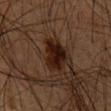Q: How was this image acquired?
A: 15 mm crop, total-body photography
Q: Patient demographics?
A: male, in their mid- to late 60s
Q: What is the anatomic site?
A: the chest